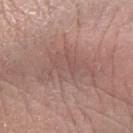Impression:
No biopsy was performed on this lesion — it was imaged during a full skin examination and was not determined to be concerning.
Clinical summary:
Captured under white-light illumination. The lesion's longest dimension is about 6.5 mm. The subject is a male roughly 60 years of age. Cropped from a whole-body photographic skin survey; the tile spans about 15 mm. On the left forearm. The total-body-photography lesion software estimated a footprint of about 15 mm², an eccentricity of roughly 0.8, and a shape-asymmetry score of about 0.55 (0 = symmetric). It also reported a mean CIELAB color near L≈53 a*≈19 b*≈22 and a lesion-to-skin contrast of about 5 (normalized; higher = more distinct). It also reported a border-irregularity index near 9/10, a color-variation rating of about 2.5/10, and peripheral color asymmetry of about 0.5. The analysis additionally found a nevus-likeness score of about 0/100 and a detector confidence of about 65 out of 100 that the crop contains a lesion.